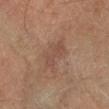Notes:
• biopsy status: imaged on a skin check; not biopsied
• image: 15 mm crop, total-body photography
• diameter: ≈5 mm
• lighting: cross-polarized
• automated metrics: an area of roughly 9.5 mm² and two-axis asymmetry of about 0.35; a mean CIELAB color near L≈43 a*≈17 b*≈25, a lesion–skin lightness drop of about 6, and a lesion-to-skin contrast of about 4.5 (normalized; higher = more distinct)
• anatomic site: the right forearm
• subject: male, in their 60s